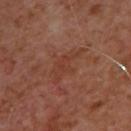<lesion>
<lesion_size>
  <long_diameter_mm_approx>5.0</long_diameter_mm_approx>
</lesion_size>
<patient>
  <sex>male</sex>
  <age_approx>50</age_approx>
</patient>
<site>upper back</site>
<image>
  <source>total-body photography crop</source>
  <field_of_view_mm>15</field_of_view_mm>
</image>
</lesion>